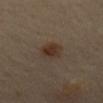Q: Is there a histopathology result?
A: catalogued during a skin exam; not biopsied
Q: What are the patient's age and sex?
A: male, in their mid- to late 60s
Q: How large is the lesion?
A: ~3.5 mm (longest diameter)
Q: What is the anatomic site?
A: the mid back
Q: What lighting was used for the tile?
A: cross-polarized illumination
Q: How was this image acquired?
A: ~15 mm crop, total-body skin-cancer survey